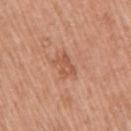workup — no biopsy performed (imaged during a skin exam)
tile lighting — white-light
lesion diameter — about 3 mm
anatomic site — the right upper arm
image source — ~15 mm crop, total-body skin-cancer survey
subject — male, roughly 60 years of age